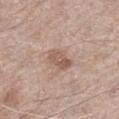Q: Was this lesion biopsied?
A: catalogued during a skin exam; not biopsied
Q: What are the patient's age and sex?
A: male, about 70 years old
Q: Lesion size?
A: ≈3.5 mm
Q: Automated lesion metrics?
A: an automated nevus-likeness rating near 55 out of 100 and a detector confidence of about 100 out of 100 that the crop contains a lesion
Q: Lesion location?
A: the left thigh
Q: What lighting was used for the tile?
A: white-light
Q: How was this image acquired?
A: ~15 mm crop, total-body skin-cancer survey